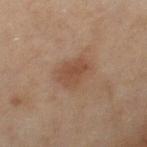follow-up — total-body-photography surveillance lesion; no biopsy | imaging modality — ~15 mm crop, total-body skin-cancer survey | subject — female, aged 58–62 | site — the leg | size — ~3.5 mm (longest diameter) | TBP lesion metrics — an average lesion color of about L≈43 a*≈18 b*≈27 (CIELAB), roughly 7 lightness units darker than nearby skin, and a normalized lesion–skin contrast near 6.5; a border-irregularity rating of about 2.5/10 and a within-lesion color-variation index near 2/10.